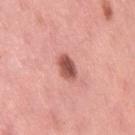Part of a total-body skin-imaging series; this lesion was reviewed on a skin check and was not flagged for biopsy. This is a white-light tile. On the right thigh. Automated tile analysis of the lesion measured a mean CIELAB color near L≈54 a*≈29 b*≈27 and a lesion-to-skin contrast of about 10 (normalized; higher = more distinct). The software also gave a border-irregularity rating of about 1.5/10, a color-variation rating of about 3.5/10, and radial color variation of about 1.5. The software also gave a nevus-likeness score of about 95/100 and a lesion-detection confidence of about 100/100. A close-up tile cropped from a whole-body skin photograph, about 15 mm across. About 3 mm across. A female patient, about 50 years old.